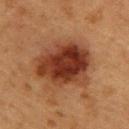{
  "biopsy_status": "not biopsied; imaged during a skin examination",
  "lesion_size": {
    "long_diameter_mm_approx": 6.5
  },
  "site": "upper back",
  "lighting": "cross-polarized",
  "image": {
    "source": "total-body photography crop",
    "field_of_view_mm": 15
  },
  "patient": {
    "sex": "male",
    "age_approx": 55
  }
}